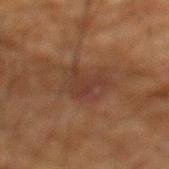Impression: Part of a total-body skin-imaging series; this lesion was reviewed on a skin check and was not flagged for biopsy. Clinical summary: The tile uses cross-polarized illumination. A 15 mm close-up extracted from a 3D total-body photography capture. The lesion is on the mid back. The patient is a male aged approximately 60.